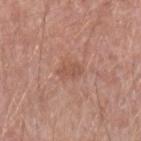Imaged during a routine full-body skin examination; the lesion was not biopsied and no histopathology is available.
This is a white-light tile.
Measured at roughly 3 mm in maximum diameter.
Cropped from a whole-body photographic skin survey; the tile spans about 15 mm.
On the arm.
A male patient, aged around 60.